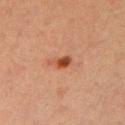No biopsy was performed on this lesion — it was imaged during a full skin examination and was not determined to be concerning. A close-up tile cropped from a whole-body skin photograph, about 15 mm across. The patient is a male about 40 years old. On the left upper arm.